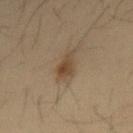The lesion was tiled from a total-body skin photograph and was not biopsied.
Imaged with cross-polarized lighting.
On the mid back.
The total-body-photography lesion software estimated a footprint of about 5.5 mm² and an eccentricity of roughly 0.9. The software also gave border irregularity of about 3.5 on a 0–10 scale and a peripheral color-asymmetry measure near 1. The software also gave a classifier nevus-likeness of about 90/100.
A roughly 15 mm field-of-view crop from a total-body skin photograph.
A male patient, approximately 35 years of age.